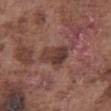The lesion was tiled from a total-body skin photograph and was not biopsied. The subject is a male in their mid-70s. From the front of the torso. The tile uses white-light illumination. A region of skin cropped from a whole-body photographic capture, roughly 15 mm wide. Measured at roughly 4 mm in maximum diameter.